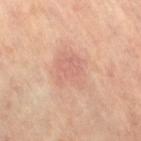| field | value |
|---|---|
| follow-up | imaged on a skin check; not biopsied |
| location | the left leg |
| imaging modality | ~15 mm crop, total-body skin-cancer survey |
| diameter | about 5 mm |
| image-analysis metrics | an average lesion color of about L≈63 a*≈22 b*≈28 (CIELAB), a lesion–skin lightness drop of about 7, and a normalized lesion–skin contrast near 4.5 |
| patient | female, about 65 years old |
| tile lighting | cross-polarized illumination |